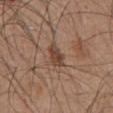Clinical impression: The lesion was photographed on a routine skin check and not biopsied; there is no pathology result. Acquisition and patient details: The subject is a male roughly 75 years of age. Measured at roughly 3 mm in maximum diameter. Cropped from a whole-body photographic skin survey; the tile spans about 15 mm. Located on the abdomen. This is a white-light tile.